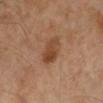The lesion was tiled from a total-body skin photograph and was not biopsied.
The lesion-visualizer software estimated an eccentricity of roughly 0.75. The software also gave a lesion color around L≈43 a*≈21 b*≈32 in CIELAB, about 9 CIELAB-L* units darker than the surrounding skin, and a normalized lesion–skin contrast near 7.5. The analysis additionally found a border-irregularity rating of about 2/10.
A close-up tile cropped from a whole-body skin photograph, about 15 mm across.
The tile uses cross-polarized illumination.
Longest diameter approximately 4 mm.
From the left lower leg.
A male patient, about 55 years old.